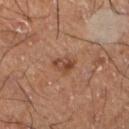Part of a total-body skin-imaging series; this lesion was reviewed on a skin check and was not flagged for biopsy.
Measured at roughly 3 mm in maximum diameter.
Captured under cross-polarized illumination.
A close-up tile cropped from a whole-body skin photograph, about 15 mm across.
Located on the left lower leg.
A male patient, in their 60s.